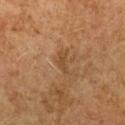biopsy status — total-body-photography surveillance lesion; no biopsy
imaging modality — 15 mm crop, total-body photography
subject — female, aged 58–62
tile lighting — cross-polarized
size — ≈2.5 mm
body site — the left upper arm